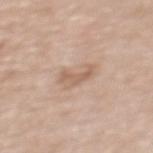| key | value |
|---|---|
| notes | no biopsy performed (imaged during a skin exam) |
| tile lighting | white-light |
| image | ~15 mm crop, total-body skin-cancer survey |
| location | the upper back |
| size | about 3.5 mm |
| subject | female, aged 63 to 67 |